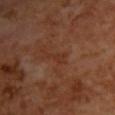• notes — imaged on a skin check; not biopsied
• acquisition — ~15 mm tile from a whole-body skin photo
• diameter — ≈2.5 mm
• patient — female
• image-analysis metrics — an area of roughly 2.5 mm², an outline eccentricity of about 0.9 (0 = round, 1 = elongated), and a shape-asymmetry score of about 0.45 (0 = symmetric); a nevus-likeness score of about 0/100 and a lesion-detection confidence of about 95/100
• body site — the upper back